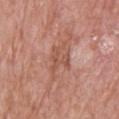Q: Was a biopsy performed?
A: imaged on a skin check; not biopsied
Q: Patient demographics?
A: female, approximately 65 years of age
Q: Lesion location?
A: the back
Q: What is the imaging modality?
A: 15 mm crop, total-body photography